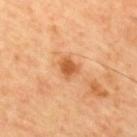{"biopsy_status": "not biopsied; imaged during a skin examination", "site": "back", "automated_metrics": {"vs_skin_darker_L": 11.0, "vs_skin_contrast_norm": 8.0, "lesion_detection_confidence_0_100": 100}, "patient": {"sex": "male", "age_approx": 60}, "image": {"source": "total-body photography crop", "field_of_view_mm": 15}, "lighting": "cross-polarized"}